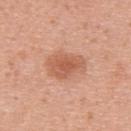{"biopsy_status": "not biopsied; imaged during a skin examination", "patient": {"sex": "female", "age_approx": 30}, "automated_metrics": {"nevus_likeness_0_100": 90, "lesion_detection_confidence_0_100": 100}, "image": {"source": "total-body photography crop", "field_of_view_mm": 15}, "lighting": "white-light", "site": "upper back", "lesion_size": {"long_diameter_mm_approx": 4.5}}